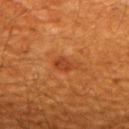Q: Patient demographics?
A: male, aged 58 to 62
Q: What kind of image is this?
A: total-body-photography crop, ~15 mm field of view
Q: How was the tile lit?
A: cross-polarized
Q: Where on the body is the lesion?
A: the upper back